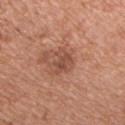Findings:
– biopsy status · total-body-photography surveillance lesion; no biopsy